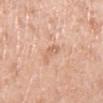No biopsy was performed on this lesion — it was imaged during a full skin examination and was not determined to be concerning.
This image is a 15 mm lesion crop taken from a total-body photograph.
About 3.5 mm across.
The lesion is located on the right lower leg.
A female subject aged 68–72.
Imaged with white-light lighting.